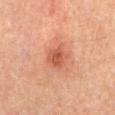Q: Is there a histopathology result?
A: no biopsy performed (imaged during a skin exam)
Q: How large is the lesion?
A: ~2.5 mm (longest diameter)
Q: What kind of image is this?
A: ~15 mm crop, total-body skin-cancer survey
Q: Who is the patient?
A: male, aged 63–67
Q: How was the tile lit?
A: cross-polarized
Q: What did automated image analysis measure?
A: border irregularity of about 2.5 on a 0–10 scale and radial color variation of about 1.5; an automated nevus-likeness rating near 55 out of 100 and a detector confidence of about 100 out of 100 that the crop contains a lesion
Q: Lesion location?
A: the mid back